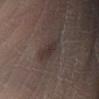follow-up: total-body-photography surveillance lesion; no biopsy | image source: ~15 mm crop, total-body skin-cancer survey | diameter: about 3.5 mm | anatomic site: the right lower leg | patient: male, aged 63 to 67 | lighting: white-light.